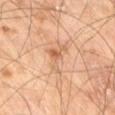notes: catalogued during a skin exam; not biopsied
diameter: ~8.5 mm (longest diameter)
lighting: cross-polarized
automated lesion analysis: a footprint of about 13 mm², an outline eccentricity of about 0.95 (0 = round, 1 = elongated), and a shape-asymmetry score of about 0.55 (0 = symmetric)
subject: male, aged 68–72
location: the right thigh
image: ~15 mm tile from a whole-body skin photo